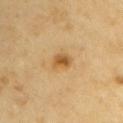Clinical impression:
No biopsy was performed on this lesion — it was imaged during a full skin examination and was not determined to be concerning.
Acquisition and patient details:
A lesion tile, about 15 mm wide, cut from a 3D total-body photograph. Longest diameter approximately 2.5 mm. Located on the left upper arm. The patient is a male about 60 years old.